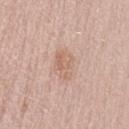Assessment:
No biopsy was performed on this lesion — it was imaged during a full skin examination and was not determined to be concerning.
Background:
A 15 mm crop from a total-body photograph taken for skin-cancer surveillance. Located on the lower back. A female patient, about 60 years old.